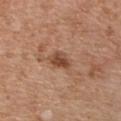No biopsy was performed on this lesion — it was imaged during a full skin examination and was not determined to be concerning. A region of skin cropped from a whole-body photographic capture, roughly 15 mm wide. The lesion's longest dimension is about 3 mm. Imaged with white-light lighting. On the chest. The subject is a male roughly 65 years of age.